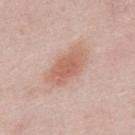| feature | finding |
|---|---|
| follow-up | no biopsy performed (imaged during a skin exam) |
| imaging modality | ~15 mm tile from a whole-body skin photo |
| anatomic site | the upper back |
| patient | male, roughly 25 years of age |